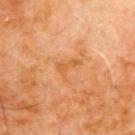{"biopsy_status": "not biopsied; imaged during a skin examination", "lighting": "cross-polarized", "image": {"source": "total-body photography crop", "field_of_view_mm": 15}, "patient": {"sex": "male", "age_approx": 70}, "lesion_size": {"long_diameter_mm_approx": 3.0}, "site": "chest", "automated_metrics": {"color_variation_0_10": 0.5, "peripheral_color_asymmetry": 0.0, "nevus_likeness_0_100": 0, "lesion_detection_confidence_0_100": 100}}